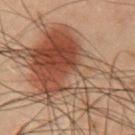Q: What is the anatomic site?
A: the chest
Q: Patient demographics?
A: male, aged approximately 55
Q: Illumination type?
A: cross-polarized illumination
Q: How was this image acquired?
A: 15 mm crop, total-body photography
Q: Lesion size?
A: ≈13.5 mm
Q: What did automated image analysis measure?
A: a lesion area of about 60 mm², an eccentricity of roughly 0.85, and a shape-asymmetry score of about 0.55 (0 = symmetric); a lesion color around L≈39 a*≈17 b*≈25 in CIELAB, about 10 CIELAB-L* units darker than the surrounding skin, and a lesion-to-skin contrast of about 8.5 (normalized; higher = more distinct)